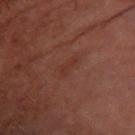This lesion was catalogued during total-body skin photography and was not selected for biopsy. A 15 mm close-up tile from a total-body photography series done for melanoma screening. A female subject, roughly 65 years of age. The lesion is located on the left forearm.